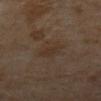Notes:
– notes — no biopsy performed (imaged during a skin exam)
– image source — total-body-photography crop, ~15 mm field of view
– tile lighting — cross-polarized illumination
– lesion diameter — ~3 mm (longest diameter)
– patient — male, roughly 65 years of age